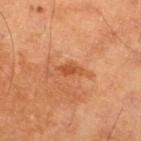Clinical impression:
The lesion was photographed on a routine skin check and not biopsied; there is no pathology result.
Clinical summary:
A male patient, about 60 years old. About 3 mm across. An algorithmic analysis of the crop reported a footprint of about 3.5 mm², an outline eccentricity of about 0.9 (0 = round, 1 = elongated), and a symmetry-axis asymmetry near 0.3. The analysis additionally found a lesion–skin lightness drop of about 9 and a normalized lesion–skin contrast near 6.5. And it measured a border-irregularity index near 3/10, a color-variation rating of about 1.5/10, and peripheral color asymmetry of about 0.5. This image is a 15 mm lesion crop taken from a total-body photograph. Located on the left lower leg.